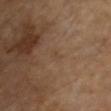Assessment:
The lesion was photographed on a routine skin check and not biopsied; there is no pathology result.
Background:
Longest diameter approximately 1 mm. A close-up tile cropped from a whole-body skin photograph, about 15 mm across. Automated tile analysis of the lesion measured a lesion color around L≈42 a*≈16 b*≈29 in CIELAB and a normalized border contrast of about 3.5. And it measured a border-irregularity index near 2/10, internal color variation of about 0 on a 0–10 scale, and peripheral color asymmetry of about 0. The software also gave an automated nevus-likeness rating near 0 out of 100 and a lesion-detection confidence of about 100/100. A female patient in their mid- to late 60s. The lesion is on the chest. This is a cross-polarized tile.